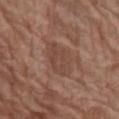Recorded during total-body skin imaging; not selected for excision or biopsy. Measured at roughly 5 mm in maximum diameter. An algorithmic analysis of the crop reported a mean CIELAB color near L≈44 a*≈19 b*≈25 and a lesion-to-skin contrast of about 5.5 (normalized; higher = more distinct). Located on the abdomen. A lesion tile, about 15 mm wide, cut from a 3D total-body photograph. A female patient about 75 years old.